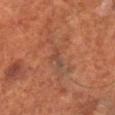biopsy status = catalogued during a skin exam; not biopsied
lighting = cross-polarized
location = the right lower leg
acquisition = 15 mm crop, total-body photography
patient = male, roughly 70 years of age